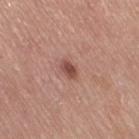biopsy status = imaged on a skin check; not biopsied
site = the right thigh
acquisition = 15 mm crop, total-body photography
patient = female, roughly 65 years of age